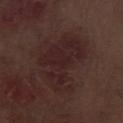Captured during whole-body skin photography for melanoma surveillance; the lesion was not biopsied. From the leg. The lesion's longest dimension is about 7.5 mm. The subject is a male aged around 70. A lesion tile, about 15 mm wide, cut from a 3D total-body photograph. The tile uses white-light illumination.